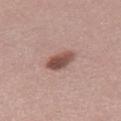Q: Was this lesion biopsied?
A: catalogued during a skin exam; not biopsied
Q: Where on the body is the lesion?
A: the right thigh
Q: What is the lesion's diameter?
A: ≈4 mm
Q: What are the patient's age and sex?
A: female, roughly 40 years of age
Q: What is the imaging modality?
A: 15 mm crop, total-body photography
Q: Illumination type?
A: white-light illumination